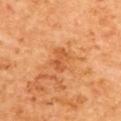follow-up: total-body-photography surveillance lesion; no biopsy
TBP lesion metrics: a mean CIELAB color near L≈59 a*≈31 b*≈46, a lesion–skin lightness drop of about 8, and a normalized lesion–skin contrast near 5.5
location: the upper back
tile lighting: cross-polarized
lesion diameter: ~3 mm (longest diameter)
patient: female
imaging modality: ~15 mm tile from a whole-body skin photo